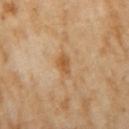| feature | finding |
|---|---|
| workup | imaged on a skin check; not biopsied |
| lighting | cross-polarized |
| subject | female, aged approximately 70 |
| diameter | ~3 mm (longest diameter) |
| image-analysis metrics | an outline eccentricity of about 0.8 (0 = round, 1 = elongated); an average lesion color of about L≈57 a*≈20 b*≈40 (CIELAB), about 9 CIELAB-L* units darker than the surrounding skin, and a normalized border contrast of about 7; border irregularity of about 2 on a 0–10 scale, a within-lesion color-variation index near 3/10, and a peripheral color-asymmetry measure near 1; a nevus-likeness score of about 50/100 and lesion-presence confidence of about 100/100 |
| body site | the right upper arm |
| image source | ~15 mm tile from a whole-body skin photo |